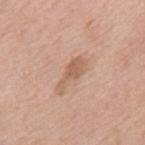Clinical impression: The lesion was photographed on a routine skin check and not biopsied; there is no pathology result. Clinical summary: A 15 mm close-up tile from a total-body photography series done for melanoma screening. A male subject, aged 78–82. Approximately 4.5 mm at its widest. Located on the mid back.